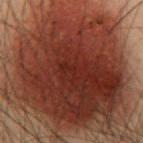A male subject aged 53–57. Located on the front of the torso. The lesion's longest dimension is about 13.5 mm. A roughly 15 mm field-of-view crop from a total-body skin photograph.